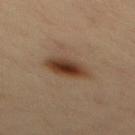notes = catalogued during a skin exam; not biopsied | body site = the mid back | imaging modality = ~15 mm crop, total-body skin-cancer survey | image-analysis metrics = a border-irregularity index near 1.5/10, a color-variation rating of about 7/10, and peripheral color asymmetry of about 2 | patient = male, about 35 years old | diameter = about 4.5 mm | tile lighting = cross-polarized.